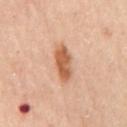Part of a total-body skin-imaging series; this lesion was reviewed on a skin check and was not flagged for biopsy. On the mid back. The subject is a female in their 60s. Cropped from a total-body skin-imaging series; the visible field is about 15 mm.